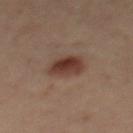Part of a total-body skin-imaging series; this lesion was reviewed on a skin check and was not flagged for biopsy. A lesion tile, about 15 mm wide, cut from a 3D total-body photograph. Imaged with cross-polarized lighting. The patient is a female roughly 45 years of age. On the mid back.